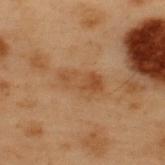Impression: Captured during whole-body skin photography for melanoma surveillance; the lesion was not biopsied. Acquisition and patient details: On the upper back. A male patient, about 55 years old. A region of skin cropped from a whole-body photographic capture, roughly 15 mm wide.